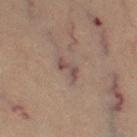| feature | finding |
|---|---|
| workup | total-body-photography surveillance lesion; no biopsy |
| illumination | cross-polarized illumination |
| location | the leg |
| image source | ~15 mm crop, total-body skin-cancer survey |
| lesion size | about 3.5 mm |
| subject | female, approximately 70 years of age |